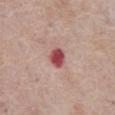Assessment: This lesion was catalogued during total-body skin photography and was not selected for biopsy.